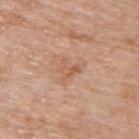Part of a total-body skin-imaging series; this lesion was reviewed on a skin check and was not flagged for biopsy.
A close-up tile cropped from a whole-body skin photograph, about 15 mm across.
This is a white-light tile.
A male subject aged approximately 75.
Located on the upper back.